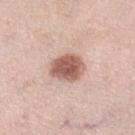Q: Was this lesion biopsied?
A: catalogued during a skin exam; not biopsied
Q: What lighting was used for the tile?
A: white-light illumination
Q: Lesion location?
A: the left lower leg
Q: What kind of image is this?
A: ~15 mm tile from a whole-body skin photo
Q: Patient demographics?
A: female, aged 63 to 67